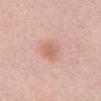Assessment: Captured during whole-body skin photography for melanoma surveillance; the lesion was not biopsied.